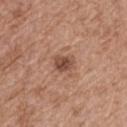| key | value |
|---|---|
| tile lighting | white-light |
| imaging modality | 15 mm crop, total-body photography |
| lesion size | ~2.5 mm (longest diameter) |
| TBP lesion metrics | an area of roughly 4 mm², a shape eccentricity near 0.7, and two-axis asymmetry of about 0.3; an average lesion color of about L≈47 a*≈22 b*≈28 (CIELAB), a lesion–skin lightness drop of about 12, and a normalized border contrast of about 9; border irregularity of about 2.5 on a 0–10 scale, internal color variation of about 3 on a 0–10 scale, and a peripheral color-asymmetry measure near 1; a nevus-likeness score of about 65/100 and lesion-presence confidence of about 100/100 |
| subject | female, approximately 75 years of age |
| site | the left thigh |